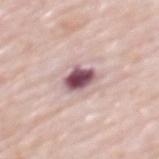Clinical impression: Imaged during a routine full-body skin examination; the lesion was not biopsied and no histopathology is available. Background: The tile uses white-light illumination. A male subject, roughly 80 years of age. A lesion tile, about 15 mm wide, cut from a 3D total-body photograph. Located on the mid back.